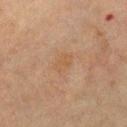biopsy status = no biopsy performed (imaged during a skin exam)
tile lighting = cross-polarized illumination
body site = the chest
subject = male, aged 68 to 72
imaging modality = ~15 mm crop, total-body skin-cancer survey
size = ≈3 mm